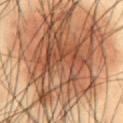{
  "biopsy_status": "not biopsied; imaged during a skin examination",
  "patient": {
    "sex": "male",
    "age_approx": 55
  },
  "site": "abdomen",
  "lesion_size": {
    "long_diameter_mm_approx": 17.0
  },
  "automated_metrics": {
    "area_mm2_approx": 120.0,
    "eccentricity": 0.75,
    "shape_asymmetry": 0.35,
    "cielab_L": 45,
    "cielab_a": 17,
    "cielab_b": 28,
    "vs_skin_darker_L": 13.0,
    "vs_skin_contrast_norm": 10.0,
    "border_irregularity_0_10": 6.5,
    "color_variation_0_10": 8.5
  },
  "image": {
    "source": "total-body photography crop",
    "field_of_view_mm": 15
  }
}